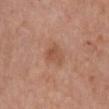Q: Was this lesion biopsied?
A: imaged on a skin check; not biopsied
Q: How large is the lesion?
A: ~2.5 mm (longest diameter)
Q: What is the imaging modality?
A: ~15 mm crop, total-body skin-cancer survey
Q: What did automated image analysis measure?
A: a lesion color around L≈52 a*≈23 b*≈32 in CIELAB, a lesion–skin lightness drop of about 8, and a normalized border contrast of about 6
Q: Patient demographics?
A: female, aged 63–67
Q: How was the tile lit?
A: white-light illumination
Q: Lesion location?
A: the chest